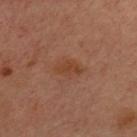Clinical impression:
This lesion was catalogued during total-body skin photography and was not selected for biopsy.
Clinical summary:
A lesion tile, about 15 mm wide, cut from a 3D total-body photograph. Approximately 3 mm at its widest. A female patient aged 53 to 57. The lesion is located on the chest. Imaged with cross-polarized lighting. The lesion-visualizer software estimated a lesion area of about 5 mm² and an outline eccentricity of about 0.8 (0 = round, 1 = elongated). It also reported a mean CIELAB color near L≈34 a*≈19 b*≈27 and a normalized lesion–skin contrast near 6.5. The software also gave a classifier nevus-likeness of about 5/100 and lesion-presence confidence of about 100/100.